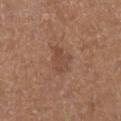Q: What lighting was used for the tile?
A: white-light
Q: What is the lesion's diameter?
A: ~3 mm (longest diameter)
Q: Lesion location?
A: the right lower leg
Q: What are the patient's age and sex?
A: male, aged 73 to 77
Q: What is the imaging modality?
A: ~15 mm tile from a whole-body skin photo